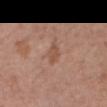Findings:
* notes · catalogued during a skin exam; not biopsied
* image · ~15 mm crop, total-body skin-cancer survey
* patient · female, aged 73 to 77
* location · the chest